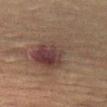Assessment: This lesion was catalogued during total-body skin photography and was not selected for biopsy.